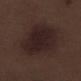Q: What is the lesion's diameter?
A: about 7 mm
Q: What did automated image analysis measure?
A: an outline eccentricity of about 0.7 (0 = round, 1 = elongated)
Q: What lighting was used for the tile?
A: white-light illumination
Q: How was this image acquired?
A: ~15 mm tile from a whole-body skin photo
Q: Who is the patient?
A: male, aged approximately 70
Q: Lesion location?
A: the leg